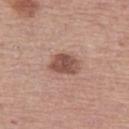notes — catalogued during a skin exam; not biopsied | subject — female, approximately 50 years of age | body site — the leg | lighting — white-light illumination | imaging modality — ~15 mm crop, total-body skin-cancer survey | lesion size — about 4 mm | image-analysis metrics — a lesion area of about 8 mm² and an eccentricity of roughly 0.7; roughly 13 lightness units darker than nearby skin and a lesion-to-skin contrast of about 9 (normalized; higher = more distinct).